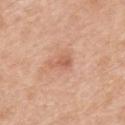| feature | finding |
|---|---|
| notes | catalogued during a skin exam; not biopsied |
| illumination | white-light |
| imaging modality | 15 mm crop, total-body photography |
| anatomic site | the mid back |
| size | ≈3 mm |
| patient | male, aged 68–72 |